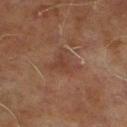Part of a total-body skin-imaging series; this lesion was reviewed on a skin check and was not flagged for biopsy.
From the right lower leg.
Automated image analysis of the tile measured an area of roughly 7 mm², an outline eccentricity of about 0.4 (0 = round, 1 = elongated), and two-axis asymmetry of about 0.55. It also reported a lesion color around L≈33 a*≈17 b*≈23 in CIELAB, roughly 5 lightness units darker than nearby skin, and a normalized lesion–skin contrast near 5. It also reported a color-variation rating of about 2/10 and radial color variation of about 0.5.
Cropped from a total-body skin-imaging series; the visible field is about 15 mm.
Imaged with cross-polarized lighting.
The patient is a male aged approximately 70.